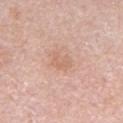Captured during whole-body skin photography for melanoma surveillance; the lesion was not biopsied. Measured at roughly 2.5 mm in maximum diameter. A male subject, in their 60s. On the abdomen. This image is a 15 mm lesion crop taken from a total-body photograph. Imaged with white-light lighting.